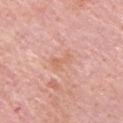Imaged during a routine full-body skin examination; the lesion was not biopsied and no histopathology is available. The tile uses white-light illumination. An algorithmic analysis of the crop reported an area of roughly 4 mm². The analysis additionally found roughly 6 lightness units darker than nearby skin. And it measured a classifier nevus-likeness of about 0/100 and lesion-presence confidence of about 100/100. The lesion is on the upper back. Measured at roughly 3 mm in maximum diameter. A lesion tile, about 15 mm wide, cut from a 3D total-body photograph. The subject is a male aged around 65.